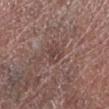Notes:
– biopsy status: catalogued during a skin exam; not biopsied
– subject: male, aged 63 to 67
– site: the left lower leg
– acquisition: ~15 mm crop, total-body skin-cancer survey